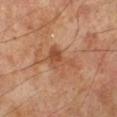lesion size=~4 mm (longest diameter) | acquisition=15 mm crop, total-body photography | site=the left lower leg | subject=male, roughly 70 years of age.